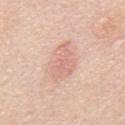<tbp_lesion>
  <biopsy_status>not biopsied; imaged during a skin examination</biopsy_status>
  <site>mid back</site>
  <patient>
    <sex>female</sex>
    <age_approx>70</age_approx>
  </patient>
  <image>
    <source>total-body photography crop</source>
    <field_of_view_mm>15</field_of_view_mm>
  </image>
  <lighting>white-light</lighting>
  <automated_metrics>
    <border_irregularity_0_10>4.0</border_irregularity_0_10>
    <color_variation_0_10>2.5</color_variation_0_10>
    <peripheral_color_asymmetry>1.0</peripheral_color_asymmetry>
    <nevus_likeness_0_100>5</nevus_likeness_0_100>
    <lesion_detection_confidence_0_100>100</lesion_detection_confidence_0_100>
  </automated_metrics>
  <lesion_size>
    <long_diameter_mm_approx>5.0</long_diameter_mm_approx>
  </lesion_size>
</tbp_lesion>